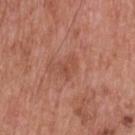{"biopsy_status": "not biopsied; imaged during a skin examination", "lighting": "white-light", "lesion_size": {"long_diameter_mm_approx": 2.5}, "site": "upper back", "automated_metrics": {"border_irregularity_0_10": 3.5, "color_variation_0_10": 1.0}, "image": {"source": "total-body photography crop", "field_of_view_mm": 15}, "patient": {"sex": "male", "age_approx": 60}}